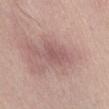Clinical impression:
This lesion was catalogued during total-body skin photography and was not selected for biopsy.
Context:
Longest diameter approximately 3 mm. An algorithmic analysis of the crop reported a border-irregularity rating of about 2.5/10, internal color variation of about 1.5 on a 0–10 scale, and radial color variation of about 0.5. The software also gave a nevus-likeness score of about 0/100 and lesion-presence confidence of about 100/100. The tile uses white-light illumination. Cropped from a whole-body photographic skin survey; the tile spans about 15 mm. A male patient, approximately 55 years of age. The lesion is located on the abdomen.